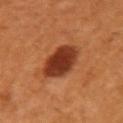{
  "biopsy_status": "not biopsied; imaged during a skin examination"
}